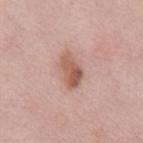{
  "image": {
    "source": "total-body photography crop",
    "field_of_view_mm": 15
  },
  "site": "front of the torso",
  "lesion_size": {
    "long_diameter_mm_approx": 4.0
  },
  "patient": {
    "sex": "female",
    "age_approx": 40
  }
}